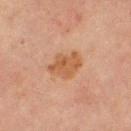  biopsy_status: not biopsied; imaged during a skin examination
  lighting: cross-polarized
  automated_metrics:
    shape_asymmetry: 0.25
    nevus_likeness_0_100: 25
    lesion_detection_confidence_0_100: 100
  image:
    source: total-body photography crop
    field_of_view_mm: 15
  lesion_size:
    long_diameter_mm_approx: 3.5
  patient:
    sex: female
    age_approx: 70
  site: right thigh